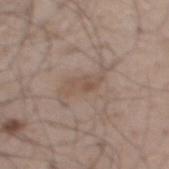Q: Was a biopsy performed?
A: no biopsy performed (imaged during a skin exam)
Q: Lesion size?
A: ≈3.5 mm
Q: How was this image acquired?
A: 15 mm crop, total-body photography
Q: Patient demographics?
A: male, in their 50s
Q: What is the anatomic site?
A: the mid back